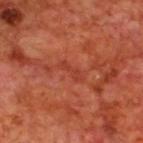This lesion was catalogued during total-body skin photography and was not selected for biopsy.
A male subject, aged 68–72.
The tile uses cross-polarized illumination.
The lesion's longest dimension is about 3 mm.
The lesion is on the upper back.
The total-body-photography lesion software estimated a border-irregularity rating of about 4/10, internal color variation of about 0 on a 0–10 scale, and a peripheral color-asymmetry measure near 0. The analysis additionally found a detector confidence of about 95 out of 100 that the crop contains a lesion.
A lesion tile, about 15 mm wide, cut from a 3D total-body photograph.